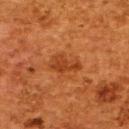The lesion was tiled from a total-body skin photograph and was not biopsied.
On the upper back.
The recorded lesion diameter is about 3.5 mm.
A 15 mm close-up tile from a total-body photography series done for melanoma screening.
The tile uses cross-polarized illumination.
A female patient, aged approximately 50.
The total-body-photography lesion software estimated a shape eccentricity near 0.85 and two-axis asymmetry of about 0.4. The software also gave a lesion color around L≈35 a*≈26 b*≈36 in CIELAB, roughly 7 lightness units darker than nearby skin, and a lesion-to-skin contrast of about 6.5 (normalized; higher = more distinct). The software also gave a within-lesion color-variation index near 2/10. The analysis additionally found an automated nevus-likeness rating near 65 out of 100 and a lesion-detection confidence of about 100/100.